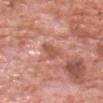workup — catalogued during a skin exam; not biopsied | patient — male, aged around 80 | image source — 15 mm crop, total-body photography | lesion diameter — about 3 mm | automated metrics — an area of roughly 3.5 mm² and a shape-asymmetry score of about 0.4 (0 = symmetric); a nevus-likeness score of about 0/100 | anatomic site — the head or neck | tile lighting — white-light illumination.